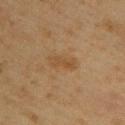Impression:
Imaged during a routine full-body skin examination; the lesion was not biopsied and no histopathology is available.
Image and clinical context:
A female patient approximately 45 years of age. The lesion is located on the upper back. This image is a 15 mm lesion crop taken from a total-body photograph. Measured at roughly 3.5 mm in maximum diameter.